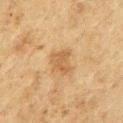{
  "biopsy_status": "not biopsied; imaged during a skin examination",
  "lighting": "cross-polarized",
  "site": "chest",
  "image": {
    "source": "total-body photography crop",
    "field_of_view_mm": 15
  },
  "lesion_size": {
    "long_diameter_mm_approx": 3.0
  },
  "patient": {
    "sex": "male",
    "age_approx": 75
  },
  "automated_metrics": {
    "area_mm2_approx": 6.5,
    "eccentricity": 0.55,
    "cielab_L": 48,
    "cielab_a": 16,
    "cielab_b": 33,
    "vs_skin_contrast_norm": 6.0,
    "border_irregularity_0_10": 3.0,
    "color_variation_0_10": 2.5,
    "peripheral_color_asymmetry": 1.0
  }
}